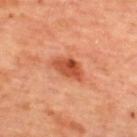<tbp_lesion>
<biopsy_status>not biopsied; imaged during a skin examination</biopsy_status>
<patient>
  <sex>female</sex>
  <age_approx>45</age_approx>
</patient>
<lighting>cross-polarized</lighting>
<site>back</site>
<lesion_size>
  <long_diameter_mm_approx>4.0</long_diameter_mm_approx>
</lesion_size>
<automated_metrics>
  <cielab_L>51</cielab_L>
  <cielab_a>33</cielab_a>
  <cielab_b>38</cielab_b>
  <vs_skin_darker_L>13.0</vs_skin_darker_L>
  <border_irregularity_0_10>2.5</border_irregularity_0_10>
  <color_variation_0_10>5.0</color_variation_0_10>
  <peripheral_color_asymmetry>1.5</peripheral_color_asymmetry>
  <lesion_detection_confidence_0_100>100</lesion_detection_confidence_0_100>
</automated_metrics>
<image>
  <source>total-body photography crop</source>
  <field_of_view_mm>15</field_of_view_mm>
</image>
</tbp_lesion>